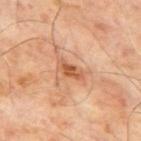<case>
<image>
  <source>total-body photography crop</source>
  <field_of_view_mm>15</field_of_view_mm>
</image>
<automated_metrics>
  <area_mm2_approx>4.5</area_mm2_approx>
  <eccentricity>0.9</eccentricity>
  <shape_asymmetry>0.35</shape_asymmetry>
  <cielab_L>55</cielab_L>
  <cielab_a>25</cielab_a>
  <cielab_b>36</cielab_b>
  <vs_skin_darker_L>11.0</vs_skin_darker_L>
  <border_irregularity_0_10>3.5</border_irregularity_0_10>
  <color_variation_0_10>4.5</color_variation_0_10>
  <peripheral_color_asymmetry>1.5</peripheral_color_asymmetry>
</automated_metrics>
<patient>
  <sex>male</sex>
  <age_approx>65</age_approx>
</patient>
<site>mid back</site>
<lesion_size>
  <long_diameter_mm_approx>3.5</long_diameter_mm_approx>
</lesion_size>
<lighting>cross-polarized</lighting>
</case>